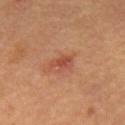biopsy status: catalogued during a skin exam; not biopsied | subject: male, aged around 65 | acquisition: total-body-photography crop, ~15 mm field of view | image-analysis metrics: an outline eccentricity of about 0.7 (0 = round, 1 = elongated) and a symmetry-axis asymmetry near 0.35; a lesion color around L≈43 a*≈25 b*≈29 in CIELAB; peripheral color asymmetry of about 1; a lesion-detection confidence of about 100/100 | lighting: cross-polarized illumination | site: the mid back | size: ~3 mm (longest diameter).